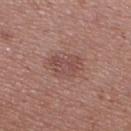Q: Is there a histopathology result?
A: catalogued during a skin exam; not biopsied
Q: How was the tile lit?
A: white-light
Q: What is the lesion's diameter?
A: ≈4 mm
Q: How was this image acquired?
A: 15 mm crop, total-body photography
Q: What are the patient's age and sex?
A: female, about 40 years old
Q: Where on the body is the lesion?
A: the right thigh